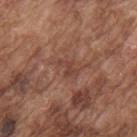tile lighting: white-light illumination
image: ~15 mm tile from a whole-body skin photo
diameter: ≈2.5 mm
subject: male, aged 73–77
location: the upper back
automated lesion analysis: a lesion area of about 3 mm², a shape eccentricity near 0.8, and a shape-asymmetry score of about 0.65 (0 = symmetric); an average lesion color of about L≈43 a*≈22 b*≈27 (CIELAB), roughly 6 lightness units darker than nearby skin, and a lesion-to-skin contrast of about 5.5 (normalized; higher = more distinct); a border-irregularity rating of about 7/10, a within-lesion color-variation index near 0/10, and radial color variation of about 0; a nevus-likeness score of about 0/100